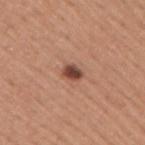Captured during whole-body skin photography for melanoma surveillance; the lesion was not biopsied. An algorithmic analysis of the crop reported a lesion area of about 3.5 mm², an eccentricity of roughly 0.7, and a symmetry-axis asymmetry near 0.25. The software also gave a lesion color around L≈46 a*≈23 b*≈28 in CIELAB, about 15 CIELAB-L* units darker than the surrounding skin, and a normalized border contrast of about 10.5. It also reported a border-irregularity index near 2/10 and radial color variation of about 1.5. It also reported a nevus-likeness score of about 95/100. The lesion is on the arm. Longest diameter approximately 2.5 mm. This image is a 15 mm lesion crop taken from a total-body photograph. A male subject aged around 45.